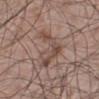Part of a total-body skin-imaging series; this lesion was reviewed on a skin check and was not flagged for biopsy.
A close-up tile cropped from a whole-body skin photograph, about 15 mm across.
On the left lower leg.
The patient is a male roughly 55 years of age.